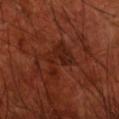Assessment: The lesion was tiled from a total-body skin photograph and was not biopsied. Background: A lesion tile, about 15 mm wide, cut from a 3D total-body photograph. A male patient aged approximately 70. The lesion is on the front of the torso.